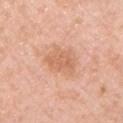  biopsy_status: not biopsied; imaged during a skin examination
  site: chest
  lighting: white-light
  image:
    source: total-body photography crop
    field_of_view_mm: 15
  patient:
    sex: male
    age_approx: 60